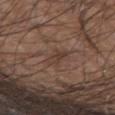Part of a total-body skin-imaging series; this lesion was reviewed on a skin check and was not flagged for biopsy. This is a white-light tile. A 15 mm close-up tile from a total-body photography series done for melanoma screening. Located on the left forearm. A male subject aged around 55. The total-body-photography lesion software estimated a lesion color around L≈38 a*≈17 b*≈23 in CIELAB and a normalized border contrast of about 6. It also reported an automated nevus-likeness rating near 0 out of 100 and lesion-presence confidence of about 65/100.